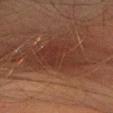{"biopsy_status": "not biopsied; imaged during a skin examination", "patient": {"sex": "female", "age_approx": 70}, "image": {"source": "total-body photography crop", "field_of_view_mm": 15}, "lighting": "cross-polarized", "lesion_size": {"long_diameter_mm_approx": 8.5}, "site": "head or neck"}